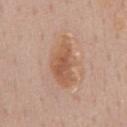Part of a total-body skin-imaging series; this lesion was reviewed on a skin check and was not flagged for biopsy. A male patient aged 58 to 62. This image is a 15 mm lesion crop taken from a total-body photograph. Located on the chest.